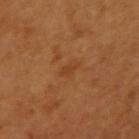Impression: The lesion was photographed on a routine skin check and not biopsied; there is no pathology result. Context: Approximately 2.5 mm at its widest. From the right upper arm. The total-body-photography lesion software estimated about 6 CIELAB-L* units darker than the surrounding skin and a lesion-to-skin contrast of about 5 (normalized; higher = more distinct). The analysis additionally found a lesion-detection confidence of about 100/100. Cropped from a total-body skin-imaging series; the visible field is about 15 mm. A female subject in their mid-50s.